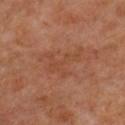notes=catalogued during a skin exam; not biopsied | diameter=~4.5 mm (longest diameter) | subject=female, aged approximately 50 | anatomic site=the chest | automated metrics=a border-irregularity rating of about 10/10 and peripheral color asymmetry of about 0; an automated nevus-likeness rating near 0 out of 100 | acquisition=15 mm crop, total-body photography.